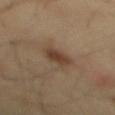Captured during whole-body skin photography for melanoma surveillance; the lesion was not biopsied. The lesion is located on the mid back. Cropped from a total-body skin-imaging series; the visible field is about 15 mm. The patient is a male roughly 40 years of age.